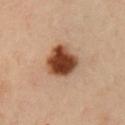  site: right upper arm
  image:
    source: total-body photography crop
    field_of_view_mm: 15
  patient:
    sex: male
    age_approx: 55